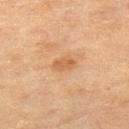biopsy status: imaged on a skin check; not biopsied | image source: ~15 mm crop, total-body skin-cancer survey | location: the left thigh | patient: female, roughly 55 years of age | illumination: cross-polarized | size: ≈3 mm | TBP lesion metrics: a shape eccentricity near 0.8; a mean CIELAB color near L≈52 a*≈20 b*≈35, roughly 8 lightness units darker than nearby skin, and a normalized border contrast of about 6; border irregularity of about 1.5 on a 0–10 scale, a within-lesion color-variation index near 3/10, and radial color variation of about 1; a classifier nevus-likeness of about 0/100 and a lesion-detection confidence of about 100/100.